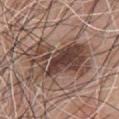biopsy status=imaged on a skin check; not biopsied | image source=total-body-photography crop, ~15 mm field of view | image-analysis metrics=a footprint of about 28 mm² | location=the chest | lighting=white-light | subject=male, aged approximately 75.